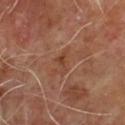workup: imaged on a skin check; not biopsied | acquisition: 15 mm crop, total-body photography | lesion size: ≈3 mm | image-analysis metrics: an area of roughly 3 mm², an outline eccentricity of about 0.9 (0 = round, 1 = elongated), and a symmetry-axis asymmetry near 0.4; roughly 6 lightness units darker than nearby skin | patient: male, in their mid- to late 60s | site: the chest.